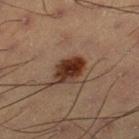workup — catalogued during a skin exam; not biopsied | subject — male, aged approximately 55 | imaging modality — ~15 mm crop, total-body skin-cancer survey | location — the right thigh.